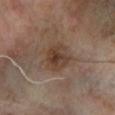{"biopsy_status": "not biopsied; imaged during a skin examination", "lesion_size": {"long_diameter_mm_approx": 3.0}, "image": {"source": "total-body photography crop", "field_of_view_mm": 15}, "patient": {"sex": "male", "age_approx": 70}, "lighting": "cross-polarized", "site": "left lower leg"}